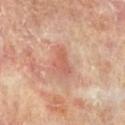Clinical impression: Part of a total-body skin-imaging series; this lesion was reviewed on a skin check and was not flagged for biopsy. Clinical summary: Automated image analysis of the tile measured a footprint of about 4.5 mm² and a symmetry-axis asymmetry near 0.45. The analysis additionally found a classifier nevus-likeness of about 0/100 and a detector confidence of about 100 out of 100 that the crop contains a lesion. A roughly 15 mm field-of-view crop from a total-body skin photograph. A female subject, roughly 75 years of age. Longest diameter approximately 3 mm. On the right lower leg.